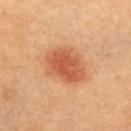Q: Was a biopsy performed?
A: no biopsy performed (imaged during a skin exam)
Q: How was this image acquired?
A: total-body-photography crop, ~15 mm field of view
Q: How was the tile lit?
A: cross-polarized
Q: What did automated image analysis measure?
A: border irregularity of about 2 on a 0–10 scale and internal color variation of about 3.5 on a 0–10 scale
Q: Lesion location?
A: the chest
Q: Who is the patient?
A: female, approximately 60 years of age